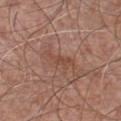This lesion was catalogued during total-body skin photography and was not selected for biopsy. The patient is a male about 65 years old. The lesion-visualizer software estimated a shape eccentricity near 0.9 and a shape-asymmetry score of about 0.45 (0 = symmetric). It also reported a border-irregularity rating of about 6/10, internal color variation of about 1 on a 0–10 scale, and a peripheral color-asymmetry measure near 0. The software also gave a detector confidence of about 95 out of 100 that the crop contains a lesion. This image is a 15 mm lesion crop taken from a total-body photograph. The recorded lesion diameter is about 4 mm. From the chest.